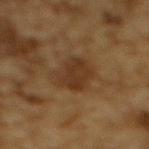Q: Was a biopsy performed?
A: catalogued during a skin exam; not biopsied
Q: What kind of image is this?
A: ~15 mm tile from a whole-body skin photo
Q: What is the anatomic site?
A: the upper back
Q: What are the patient's age and sex?
A: male, in their mid- to late 80s or older